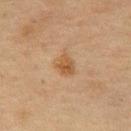Imaged during a routine full-body skin examination; the lesion was not biopsied and no histopathology is available. Automated tile analysis of the lesion measured a border-irregularity index near 2/10, internal color variation of about 3.5 on a 0–10 scale, and peripheral color asymmetry of about 1.5. The software also gave a nevus-likeness score of about 70/100 and a detector confidence of about 100 out of 100 that the crop contains a lesion. This is a cross-polarized tile. A male patient, in their mid- to late 70s. Measured at roughly 3 mm in maximum diameter. The lesion is on the abdomen. A 15 mm crop from a total-body photograph taken for skin-cancer surveillance.